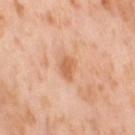The lesion was photographed on a routine skin check and not biopsied; there is no pathology result. On the leg. A female patient roughly 55 years of age. This image is a 15 mm lesion crop taken from a total-body photograph.